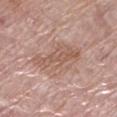Recorded during total-body skin imaging; not selected for excision or biopsy. About 6 mm across. This is a white-light tile. The patient is a female aged 58–62. A close-up tile cropped from a whole-body skin photograph, about 15 mm across. The lesion is on the left lower leg.